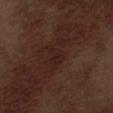The lesion was photographed on a routine skin check and not biopsied; there is no pathology result. Imaged with white-light lighting. Automated image analysis of the tile measured a lesion area of about 4 mm², an eccentricity of roughly 0.75, and a shape-asymmetry score of about 0.35 (0 = symmetric). The software also gave a border-irregularity index near 3.5/10 and a color-variation rating of about 2/10. Located on the abdomen. A roughly 15 mm field-of-view crop from a total-body skin photograph. A male patient aged 68 to 72.